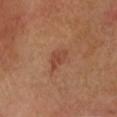follow-up = catalogued during a skin exam; not biopsied
site = the head or neck
acquisition = ~15 mm tile from a whole-body skin photo
lighting = cross-polarized
patient = female, aged 53 to 57
lesion size = ≈2.5 mm
automated lesion analysis = a lesion color around L≈46 a*≈25 b*≈32 in CIELAB, a lesion–skin lightness drop of about 8, and a normalized lesion–skin contrast near 6; a color-variation rating of about 1.5/10